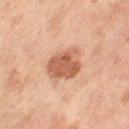Recorded during total-body skin imaging; not selected for excision or biopsy.
A female subject approximately 60 years of age.
The recorded lesion diameter is about 4 mm.
Cropped from a whole-body photographic skin survey; the tile spans about 15 mm.
Captured under cross-polarized illumination.
Automated tile analysis of the lesion measured a footprint of about 12 mm² and a shape-asymmetry score of about 0.2 (0 = symmetric). And it measured a border-irregularity index near 2/10 and radial color variation of about 1.5. And it measured a detector confidence of about 100 out of 100 that the crop contains a lesion.
The lesion is on the left thigh.